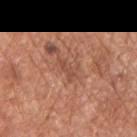Part of a total-body skin-imaging series; this lesion was reviewed on a skin check and was not flagged for biopsy.
Automated tile analysis of the lesion measured an area of roughly 5 mm² and a shape eccentricity near 0.75. It also reported a mean CIELAB color near L≈51 a*≈24 b*≈30, roughly 7 lightness units darker than nearby skin, and a normalized border contrast of about 5. It also reported a border-irregularity rating of about 4/10, internal color variation of about 3 on a 0–10 scale, and a peripheral color-asymmetry measure near 1.
The lesion is located on the left upper arm.
A roughly 15 mm field-of-view crop from a total-body skin photograph.
Captured under white-light illumination.
A male patient aged 63–67.
Measured at roughly 3 mm in maximum diameter.